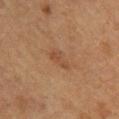biopsy_status: not biopsied; imaged during a skin examination
patient:
  sex: female
  age_approx: 70
image:
  source: total-body photography crop
  field_of_view_mm: 15
site: upper back
automated_metrics:
  cielab_L: 40
  cielab_a: 18
  cielab_b: 28
  vs_skin_darker_L: 6.0
  vs_skin_contrast_norm: 5.0